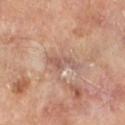{"biopsy_status": "not biopsied; imaged during a skin examination", "lesion_size": {"long_diameter_mm_approx": 4.0}, "image": {"source": "total-body photography crop", "field_of_view_mm": 15}, "site": "left lower leg", "patient": {"sex": "male", "age_approx": 65}}